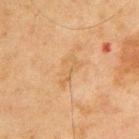patient: male, about 45 years old
acquisition: ~15 mm crop, total-body skin-cancer survey
tile lighting: cross-polarized illumination
automated lesion analysis: a lesion area of about 5 mm², a shape eccentricity near 0.9, and two-axis asymmetry of about 0.45; an automated nevus-likeness rating near 0 out of 100 and lesion-presence confidence of about 100/100
body site: the upper back
diameter: about 3.5 mm